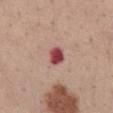Part of a total-body skin-imaging series; this lesion was reviewed on a skin check and was not flagged for biopsy.
Automated image analysis of the tile measured a lesion area of about 4.5 mm² and a shape-asymmetry score of about 0.2 (0 = symmetric). The software also gave about 17 CIELAB-L* units darker than the surrounding skin and a lesion-to-skin contrast of about 11.5 (normalized; higher = more distinct). And it measured a border-irregularity index near 1.5/10, internal color variation of about 4 on a 0–10 scale, and a peripheral color-asymmetry measure near 1. The software also gave a nevus-likeness score of about 0/100 and a detector confidence of about 100 out of 100 that the crop contains a lesion.
Longest diameter approximately 2.5 mm.
A 15 mm close-up extracted from a 3D total-body photography capture.
On the abdomen.
A male patient aged around 55.